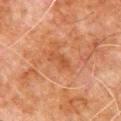The lesion was tiled from a total-body skin photograph and was not biopsied.
Measured at roughly 3 mm in maximum diameter.
On the chest.
A male subject aged 78–82.
The tile uses cross-polarized illumination.
Cropped from a total-body skin-imaging series; the visible field is about 15 mm.